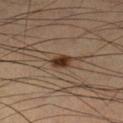* notes — total-body-photography surveillance lesion; no biopsy
* body site — the left lower leg
* lesion diameter — about 2.5 mm
* TBP lesion metrics — a footprint of about 3.5 mm²; border irregularity of about 1.5 on a 0–10 scale, a within-lesion color-variation index near 2/10, and a peripheral color-asymmetry measure near 0.5; a classifier nevus-likeness of about 100/100
* subject — male, roughly 65 years of age
* image — total-body-photography crop, ~15 mm field of view